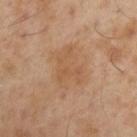lesion size: about 5 mm | illumination: cross-polarized | body site: the arm | image: ~15 mm tile from a whole-body skin photo | subject: male, roughly 55 years of age.